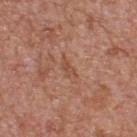The subject is a male in their mid-60s. The lesion's longest dimension is about 3 mm. Cropped from a whole-body photographic skin survey; the tile spans about 15 mm. The lesion is on the upper back. Captured under white-light illumination.